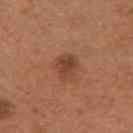No biopsy was performed on this lesion — it was imaged during a full skin examination and was not determined to be concerning. A lesion tile, about 15 mm wide, cut from a 3D total-body photograph. On the right upper arm. The subject is a female in their 30s.